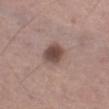Clinical impression: Recorded during total-body skin imaging; not selected for excision or biopsy. Image and clinical context: About 3.5 mm across. A male subject, approximately 75 years of age. A 15 mm crop from a total-body photograph taken for skin-cancer surveillance. Located on the left thigh. The tile uses white-light illumination.